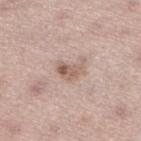No biopsy was performed on this lesion — it was imaged during a full skin examination and was not determined to be concerning. The lesion-visualizer software estimated a footprint of about 5 mm², an eccentricity of roughly 0.8, and two-axis asymmetry of about 0.4. The analysis additionally found border irregularity of about 4.5 on a 0–10 scale and a peripheral color-asymmetry measure near 2. The analysis additionally found an automated nevus-likeness rating near 40 out of 100 and lesion-presence confidence of about 100/100. Imaged with white-light lighting. Measured at roughly 3.5 mm in maximum diameter. The subject is a female roughly 50 years of age. Cropped from a total-body skin-imaging series; the visible field is about 15 mm. From the leg.